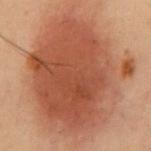biopsy_status: not biopsied; imaged during a skin examination
lesion_size:
  long_diameter_mm_approx: 15.5
site: chest
patient:
  sex: male
  age_approx: 55
image:
  source: total-body photography crop
  field_of_view_mm: 15
lighting: cross-polarized